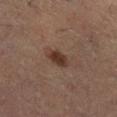Assessment: Part of a total-body skin-imaging series; this lesion was reviewed on a skin check and was not flagged for biopsy. Context: The lesion is located on the right lower leg. This image is a 15 mm lesion crop taken from a total-body photograph. The subject is a male roughly 50 years of age.